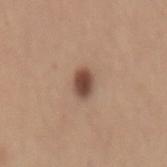Captured during whole-body skin photography for melanoma surveillance; the lesion was not biopsied. Measured at roughly 3 mm in maximum diameter. A male subject aged around 30. This is a white-light tile. Cropped from a total-body skin-imaging series; the visible field is about 15 mm. From the lower back.